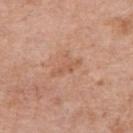workup: total-body-photography surveillance lesion; no biopsy | image-analysis metrics: a mean CIELAB color near L≈58 a*≈22 b*≈32, roughly 7 lightness units darker than nearby skin, and a normalized border contrast of about 5; a classifier nevus-likeness of about 0/100 and a detector confidence of about 100 out of 100 that the crop contains a lesion | patient: female, aged 68–72 | size: ≈4 mm | location: the right upper arm | image: ~15 mm tile from a whole-body skin photo.